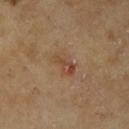<tbp_lesion>
<lesion_size>
  <long_diameter_mm_approx>3.5</long_diameter_mm_approx>
</lesion_size>
<automated_metrics>
  <area_mm2_approx>5.0</area_mm2_approx>
  <shape_asymmetry>0.3</shape_asymmetry>
  <vs_skin_darker_L>7.0</vs_skin_darker_L>
  <vs_skin_contrast_norm>6.0</vs_skin_contrast_norm>
</automated_metrics>
<lighting>cross-polarized</lighting>
<patient>
  <sex>male</sex>
  <age_approx>65</age_approx>
</patient>
<site>right lower leg</site>
<image>
  <source>total-body photography crop</source>
  <field_of_view_mm>15</field_of_view_mm>
</image>
</tbp_lesion>